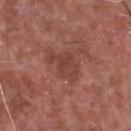Approximately 4.5 mm at its widest. From the chest. A male subject aged approximately 65. This is a white-light tile. A 15 mm close-up extracted from a 3D total-body photography capture.